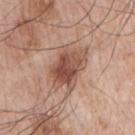Assessment: Imaged during a routine full-body skin examination; the lesion was not biopsied and no histopathology is available. Acquisition and patient details: On the right upper arm. A male subject aged 63 to 67. The total-body-photography lesion software estimated a footprint of about 16 mm², an outline eccentricity of about 0.65 (0 = round, 1 = elongated), and a shape-asymmetry score of about 0.3 (0 = symmetric). It also reported an average lesion color of about L≈52 a*≈21 b*≈27 (CIELAB) and a lesion–skin lightness drop of about 13. The software also gave an automated nevus-likeness rating near 50 out of 100. Captured under white-light illumination. Cropped from a whole-body photographic skin survey; the tile spans about 15 mm.